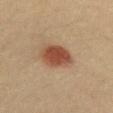Imaged during a routine full-body skin examination; the lesion was not biopsied and no histopathology is available. Approximately 4 mm at its widest. Automated tile analysis of the lesion measured a footprint of about 10 mm², a shape eccentricity near 0.65, and a shape-asymmetry score of about 0.15 (0 = symmetric). It also reported a lesion color around L≈42 a*≈21 b*≈29 in CIELAB, roughly 12 lightness units darker than nearby skin, and a normalized lesion–skin contrast near 9.5. And it measured internal color variation of about 3 on a 0–10 scale and a peripheral color-asymmetry measure near 1. Captured under cross-polarized illumination. The lesion is on the arm. A close-up tile cropped from a whole-body skin photograph, about 15 mm across. A male subject, aged 38–42.